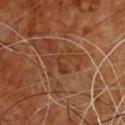Context:
A 15 mm close-up extracted from a 3D total-body photography capture. A male patient aged around 60. Located on the chest.